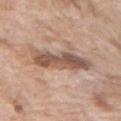A female subject, in their mid-70s.
The lesion is on the left upper arm.
The lesion's longest dimension is about 7 mm.
This image is a 15 mm lesion crop taken from a total-body photograph.
Automated tile analysis of the lesion measured an area of roughly 14 mm² and a symmetry-axis asymmetry near 0.2. The software also gave a classifier nevus-likeness of about 0/100.